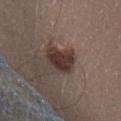The lesion was tiled from a total-body skin photograph and was not biopsied.
From the head or neck.
About 4 mm across.
Imaged with white-light lighting.
A close-up tile cropped from a whole-body skin photograph, about 15 mm across.
A female subject, aged around 25.
The total-body-photography lesion software estimated an area of roughly 10 mm², an outline eccentricity of about 0.7 (0 = round, 1 = elongated), and a shape-asymmetry score of about 0.25 (0 = symmetric). And it measured an average lesion color of about L≈34 a*≈16 b*≈19 (CIELAB), a lesion–skin lightness drop of about 12, and a lesion-to-skin contrast of about 10.5 (normalized; higher = more distinct). And it measured lesion-presence confidence of about 100/100.